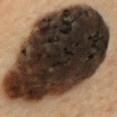A male subject, in their mid-50s. The lesion is located on the mid back. This image is a 15 mm lesion crop taken from a total-body photograph. Longest diameter approximately 16 mm.